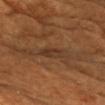Assessment:
The lesion was photographed on a routine skin check and not biopsied; there is no pathology result.
Acquisition and patient details:
Imaged with cross-polarized lighting. An algorithmic analysis of the crop reported a border-irregularity rating of about 2.5/10, a color-variation rating of about 3.5/10, and a peripheral color-asymmetry measure near 1. About 3.5 mm across. On the chest. A 15 mm close-up tile from a total-body photography series done for melanoma screening. A male patient aged around 65.